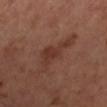Q: Was a biopsy performed?
A: no biopsy performed (imaged during a skin exam)
Q: Automated lesion metrics?
A: an area of roughly 9 mm², an eccentricity of roughly 0.85, and a shape-asymmetry score of about 0.55 (0 = symmetric); a lesion color around L≈35 a*≈21 b*≈26 in CIELAB, roughly 7 lightness units darker than nearby skin, and a normalized border contrast of about 6.5
Q: Who is the patient?
A: male, about 65 years old
Q: Where on the body is the lesion?
A: the left lower leg
Q: How was this image acquired?
A: ~15 mm crop, total-body skin-cancer survey
Q: How was the tile lit?
A: cross-polarized illumination